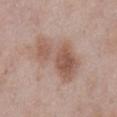Imaged with white-light lighting. A 15 mm close-up tile from a total-body photography series done for melanoma screening. The lesion is located on the front of the torso. A male subject roughly 55 years of age.